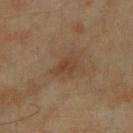Notes:
* imaging modality: ~15 mm tile from a whole-body skin photo
* anatomic site: the right forearm
* TBP lesion metrics: a footprint of about 5 mm² and a shape eccentricity near 0.8; a mean CIELAB color near L≈37 a*≈15 b*≈28, roughly 6 lightness units darker than nearby skin, and a lesion-to-skin contrast of about 5.5 (normalized; higher = more distinct); a border-irregularity rating of about 2.5/10 and radial color variation of about 1; a nevus-likeness score of about 0/100 and a detector confidence of about 100 out of 100 that the crop contains a lesion
* illumination: cross-polarized
* patient: female, roughly 60 years of age